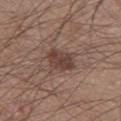Findings:
– notes · total-body-photography surveillance lesion; no biopsy
– image-analysis metrics · a lesion area of about 8.5 mm², a shape eccentricity near 0.55, and a symmetry-axis asymmetry near 0.3; a mean CIELAB color near L≈41 a*≈17 b*≈22, roughly 10 lightness units darker than nearby skin, and a normalized border contrast of about 8; an automated nevus-likeness rating near 65 out of 100
– image · ~15 mm crop, total-body skin-cancer survey
– subject · male, aged around 45
– location · the left thigh
– illumination · white-light illumination